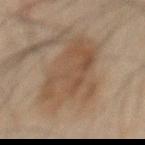The lesion was photographed on a routine skin check and not biopsied; there is no pathology result.
On the chest.
A male subject aged approximately 45.
Cropped from a whole-body photographic skin survey; the tile spans about 15 mm.